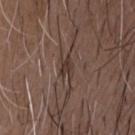The lesion was photographed on a routine skin check and not biopsied; there is no pathology result.
Measured at roughly 3 mm in maximum diameter.
Captured under white-light illumination.
On the front of the torso.
Automated image analysis of the tile measured a mean CIELAB color near L≈35 a*≈14 b*≈21, roughly 8 lightness units darker than nearby skin, and a lesion-to-skin contrast of about 7.5 (normalized; higher = more distinct). The software also gave border irregularity of about 5 on a 0–10 scale, internal color variation of about 1.5 on a 0–10 scale, and a peripheral color-asymmetry measure near 0.5. And it measured a nevus-likeness score of about 0/100 and a detector confidence of about 55 out of 100 that the crop contains a lesion.
This image is a 15 mm lesion crop taken from a total-body photograph.
A male subject, about 50 years old.